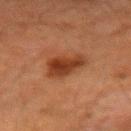notes: imaged on a skin check; not biopsied
site: the left forearm
lighting: cross-polarized illumination
imaging modality: ~15 mm crop, total-body skin-cancer survey
automated metrics: a color-variation rating of about 3.5/10 and radial color variation of about 1.5
lesion size: ≈4 mm
patient: male, in their mid- to late 60s